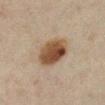– notes · catalogued during a skin exam; not biopsied
– lesion diameter · ~5 mm (longest diameter)
– image source · ~15 mm tile from a whole-body skin photo
– body site · the left lower leg
– patient · female, aged around 45